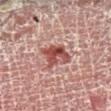Assessment: Imaged during a routine full-body skin examination; the lesion was not biopsied and no histopathology is available. Clinical summary: A 15 mm close-up tile from a total-body photography series done for melanoma screening. A male patient aged around 55. From the right lower leg.